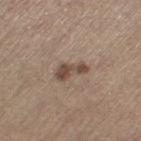Captured during whole-body skin photography for melanoma surveillance; the lesion was not biopsied.
The lesion is on the left thigh.
A 15 mm close-up extracted from a 3D total-body photography capture.
A female subject aged approximately 65.